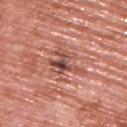follow-up = imaged on a skin check; not biopsied | anatomic site = the upper back | subject = male, aged 68–72 | image = 15 mm crop, total-body photography | image-analysis metrics = a footprint of about 7 mm², a shape eccentricity near 0.75, and a symmetry-axis asymmetry near 0.55; peripheral color asymmetry of about 3.5; an automated nevus-likeness rating near 0 out of 100 and a detector confidence of about 75 out of 100 that the crop contains a lesion | tile lighting = white-light.